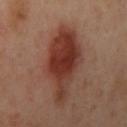notes: imaged on a skin check; not biopsied | lesion diameter: ~8.5 mm (longest diameter) | imaging modality: total-body-photography crop, ~15 mm field of view | subject: female, roughly 40 years of age | tile lighting: cross-polarized illumination | body site: the arm | automated lesion analysis: a lesion area of about 28 mm² and two-axis asymmetry of about 0.3; a border-irregularity rating of about 3.5/10, a color-variation rating of about 6/10, and radial color variation of about 2; an automated nevus-likeness rating near 100 out of 100 and a detector confidence of about 100 out of 100 that the crop contains a lesion.